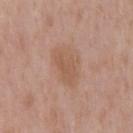Assessment: Captured during whole-body skin photography for melanoma surveillance; the lesion was not biopsied. Clinical summary: Automated tile analysis of the lesion measured a lesion area of about 8.5 mm² and a symmetry-axis asymmetry near 0.3. The software also gave a lesion color around L≈56 a*≈19 b*≈30 in CIELAB, roughly 7 lightness units darker than nearby skin, and a normalized lesion–skin contrast near 5.5. The software also gave a color-variation rating of about 2/10 and a peripheral color-asymmetry measure near 0.5. The software also gave lesion-presence confidence of about 100/100. About 4.5 mm across. A close-up tile cropped from a whole-body skin photograph, about 15 mm across. The lesion is on the mid back. The tile uses white-light illumination. A male subject roughly 55 years of age.